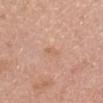<tbp_lesion>
  <biopsy_status>not biopsied; imaged during a skin examination</biopsy_status>
  <lesion_size>
    <long_diameter_mm_approx>2.0</long_diameter_mm_approx>
  </lesion_size>
  <site>head or neck</site>
  <patient>
    <sex>female</sex>
    <age_approx>40</age_approx>
  </patient>
  <lighting>white-light</lighting>
  <image>
    <source>total-body photography crop</source>
    <field_of_view_mm>15</field_of_view_mm>
  </image>
</tbp_lesion>